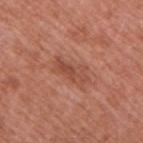Part of a total-body skin-imaging series; this lesion was reviewed on a skin check and was not flagged for biopsy. A close-up tile cropped from a whole-body skin photograph, about 15 mm across. Located on the right upper arm. A male patient, roughly 70 years of age. Automated image analysis of the tile measured a lesion area of about 7.5 mm², an outline eccentricity of about 0.9 (0 = round, 1 = elongated), and a shape-asymmetry score of about 0.3 (0 = symmetric). The analysis additionally found a lesion color around L≈49 a*≈26 b*≈31 in CIELAB, roughly 8 lightness units darker than nearby skin, and a normalized lesion–skin contrast near 5.5. The analysis additionally found border irregularity of about 4 on a 0–10 scale, internal color variation of about 4 on a 0–10 scale, and radial color variation of about 1.5.